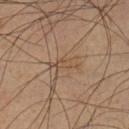biopsy status: catalogued during a skin exam; not biopsied
imaging modality: total-body-photography crop, ~15 mm field of view
patient: male, aged approximately 40
body site: the left lower leg
lighting: cross-polarized illumination
automated metrics: a footprint of about 3 mm², an eccentricity of roughly 0.95, and a symmetry-axis asymmetry near 0.5; an average lesion color of about L≈48 a*≈15 b*≈30 (CIELAB), a lesion–skin lightness drop of about 7, and a lesion-to-skin contrast of about 5.5 (normalized; higher = more distinct); a border-irregularity rating of about 6.5/10, a within-lesion color-variation index near 0/10, and radial color variation of about 0